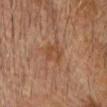Imaged during a routine full-body skin examination; the lesion was not biopsied and no histopathology is available. A lesion tile, about 15 mm wide, cut from a 3D total-body photograph. A male subject aged around 75. Imaged with cross-polarized lighting. Approximately 3 mm at its widest. On the head or neck.